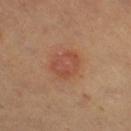Q: Is there a histopathology result?
A: total-body-photography surveillance lesion; no biopsy
Q: What is the imaging modality?
A: 15 mm crop, total-body photography
Q: Patient demographics?
A: female, approximately 50 years of age
Q: What is the anatomic site?
A: the right thigh